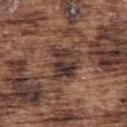Imaged during a routine full-body skin examination; the lesion was not biopsied and no histopathology is available.
A male subject in their mid-70s.
Longest diameter approximately 4 mm.
Located on the back.
A 15 mm close-up tile from a total-body photography series done for melanoma screening.
The tile uses white-light illumination.